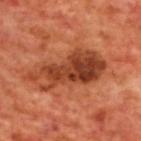follow-up: imaged on a skin check; not biopsied